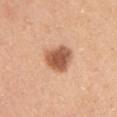Captured during whole-body skin photography for melanoma surveillance; the lesion was not biopsied. The lesion is located on the arm. Automated image analysis of the tile measured a footprint of about 9.5 mm² and an eccentricity of roughly 0.55. And it measured an average lesion color of about L≈57 a*≈25 b*≈34 (CIELAB). It also reported a border-irregularity index near 2.5/10 and a color-variation rating of about 3.5/10. The patient is a female aged 43 to 47. A roughly 15 mm field-of-view crop from a total-body skin photograph. This is a white-light tile.